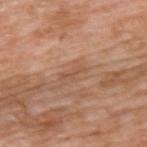biopsy_status: not biopsied; imaged during a skin examination
lighting: white-light
patient:
  sex: male
  age_approx: 60
image:
  source: total-body photography crop
  field_of_view_mm: 15
automated_metrics:
  cielab_L: 53
  cielab_a: 21
  cielab_b: 31
  vs_skin_darker_L: 7.0
  lesion_detection_confidence_0_100: 100
site: back
lesion_size:
  long_diameter_mm_approx: 3.0